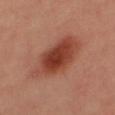Clinical impression: Captured during whole-body skin photography for melanoma surveillance; the lesion was not biopsied. Image and clinical context: A female subject. This image is a 15 mm lesion crop taken from a total-body photograph. The lesion is located on the leg.